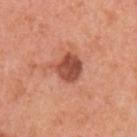Q: Was this lesion biopsied?
A: no biopsy performed (imaged during a skin exam)
Q: Automated lesion metrics?
A: a shape eccentricity near 0.3; an automated nevus-likeness rating near 30 out of 100 and lesion-presence confidence of about 100/100
Q: Patient demographics?
A: female, aged approximately 40
Q: What is the imaging modality?
A: total-body-photography crop, ~15 mm field of view
Q: Illumination type?
A: white-light
Q: Where on the body is the lesion?
A: the left upper arm